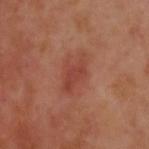Q: Was this lesion biopsied?
A: catalogued during a skin exam; not biopsied
Q: What is the lesion's diameter?
A: ~4.5 mm (longest diameter)
Q: What is the imaging modality?
A: ~15 mm crop, total-body skin-cancer survey
Q: What are the patient's age and sex?
A: male, aged around 55
Q: What lighting was used for the tile?
A: cross-polarized illumination
Q: Lesion location?
A: the upper back
Q: What did automated image analysis measure?
A: an automated nevus-likeness rating near 50 out of 100 and a lesion-detection confidence of about 100/100